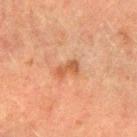Clinical impression: The lesion was tiled from a total-body skin photograph and was not biopsied. Image and clinical context: A female patient, roughly 55 years of age. From the arm. Cropped from a total-body skin-imaging series; the visible field is about 15 mm. The tile uses cross-polarized illumination. About 2.5 mm across. The lesion-visualizer software estimated an average lesion color of about L≈46 a*≈22 b*≈33 (CIELAB), a lesion–skin lightness drop of about 8, and a normalized lesion–skin contrast near 7. The analysis additionally found a nevus-likeness score of about 0/100.